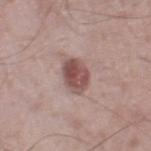Clinical impression:
No biopsy was performed on this lesion — it was imaged during a full skin examination and was not determined to be concerning.
Image and clinical context:
Measured at roughly 4 mm in maximum diameter. The tile uses white-light illumination. On the right thigh. A 15 mm close-up tile from a total-body photography series done for melanoma screening. The patient is a male aged 53–57.